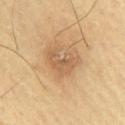  biopsy_status: not biopsied; imaged during a skin examination
  site: right upper arm
  image:
    source: total-body photography crop
    field_of_view_mm: 15
  patient:
    sex: male
    age_approx: 60